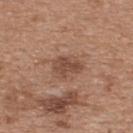Q: Was this lesion biopsied?
A: total-body-photography surveillance lesion; no biopsy
Q: Where on the body is the lesion?
A: the upper back
Q: What are the patient's age and sex?
A: female, aged approximately 40
Q: What did automated image analysis measure?
A: an eccentricity of roughly 0.7 and a symmetry-axis asymmetry near 0.25; a mean CIELAB color near L≈47 a*≈20 b*≈28, a lesion–skin lightness drop of about 10, and a normalized border contrast of about 7
Q: What lighting was used for the tile?
A: white-light illumination
Q: How was this image acquired?
A: total-body-photography crop, ~15 mm field of view
Q: What is the lesion's diameter?
A: ≈3.5 mm